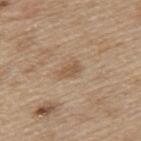Notes:
- biopsy status · imaged on a skin check; not biopsied
- diameter · ≈2.5 mm
- lighting · white-light
- image · ~15 mm tile from a whole-body skin photo
- body site · the back
- patient · male, aged around 70